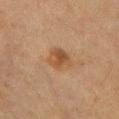biopsy status: imaged on a skin check; not biopsied | site: the chest | lighting: cross-polarized | image source: 15 mm crop, total-body photography | patient: female, aged approximately 60.